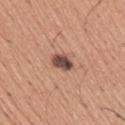notes — no biopsy performed (imaged during a skin exam)
image — total-body-photography crop, ~15 mm field of view
TBP lesion metrics — a symmetry-axis asymmetry near 0.2; border irregularity of about 2.5 on a 0–10 scale; lesion-presence confidence of about 100/100
lesion size — ~2.5 mm (longest diameter)
patient — male, approximately 45 years of age
anatomic site — the right upper arm